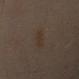patient:
  sex: male
  age_approx: 50
site: left arm
image:
  source: total-body photography crop
  field_of_view_mm: 15